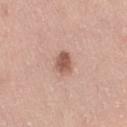follow-up — no biopsy performed (imaged during a skin exam)
tile lighting — white-light
image-analysis metrics — a border-irregularity rating of about 2/10; a classifier nevus-likeness of about 80/100
patient — female, approximately 40 years of age
location — the right thigh
imaging modality — 15 mm crop, total-body photography